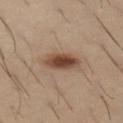Clinical impression:
Recorded during total-body skin imaging; not selected for excision or biopsy.
Context:
A male patient aged approximately 30. The tile uses cross-polarized illumination. A close-up tile cropped from a whole-body skin photograph, about 15 mm across. The lesion-visualizer software estimated internal color variation of about 4.5 on a 0–10 scale and radial color variation of about 1.5. On the left thigh.